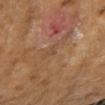Image and clinical context:
This image is a 15 mm lesion crop taken from a total-body photograph. The lesion's longest dimension is about 1 mm. A female subject, about 60 years old. The lesion is on the left arm.
Conclusion:
Histopathological examination showed an invasive squamous cell carcinoma (malignant).